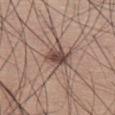Q: Was a biopsy performed?
A: no biopsy performed (imaged during a skin exam)
Q: Illumination type?
A: white-light
Q: What is the lesion's diameter?
A: ≈3.5 mm
Q: Lesion location?
A: the leg
Q: How was this image acquired?
A: ~15 mm crop, total-body skin-cancer survey
Q: Patient demographics?
A: male, approximately 60 years of age